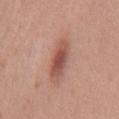notes = imaged on a skin check; not biopsied
size = ~4.5 mm (longest diameter)
illumination = white-light
image = 15 mm crop, total-body photography
automated metrics = a mean CIELAB color near L≈52 a*≈24 b*≈26, a lesion–skin lightness drop of about 12, and a normalized lesion–skin contrast near 8; a border-irregularity rating of about 2/10, internal color variation of about 5.5 on a 0–10 scale, and a peripheral color-asymmetry measure near 1.5; a classifier nevus-likeness of about 85/100
location = the back
patient = male, aged around 55